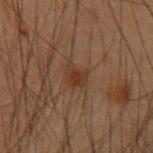Q: Is there a histopathology result?
A: no biopsy performed (imaged during a skin exam)
Q: What is the anatomic site?
A: the right forearm
Q: What kind of image is this?
A: ~15 mm crop, total-body skin-cancer survey
Q: What are the patient's age and sex?
A: male, roughly 55 years of age
Q: Lesion size?
A: ~2.5 mm (longest diameter)
Q: Automated lesion metrics?
A: an automated nevus-likeness rating near 80 out of 100 and a lesion-detection confidence of about 100/100